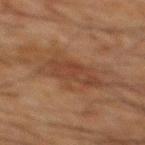notes=catalogued during a skin exam; not biopsied
anatomic site=the mid back
imaging modality=~15 mm tile from a whole-body skin photo
illumination=cross-polarized
subject=male, approximately 65 years of age